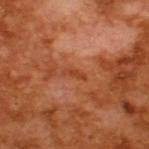Notes:
– image source · ~15 mm tile from a whole-body skin photo
– illumination · cross-polarized illumination
– subject · male, in their mid- to late 60s